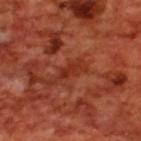Assessment: Part of a total-body skin-imaging series; this lesion was reviewed on a skin check and was not flagged for biopsy. Acquisition and patient details: A male patient aged around 70. A 15 mm crop from a total-body photograph taken for skin-cancer surveillance. This is a cross-polarized tile. The lesion is located on the upper back. Measured at roughly 3 mm in maximum diameter.